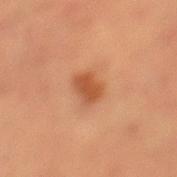<case>
<automated_metrics>
  <area_mm2_approx>6.0</area_mm2_approx>
  <eccentricity>0.7</eccentricity>
  <shape_asymmetry>0.2</shape_asymmetry>
  <cielab_L>47</cielab_L>
  <cielab_a>24</cielab_a>
  <cielab_b>35</cielab_b>
  <vs_skin_darker_L>10.0</vs_skin_darker_L>
  <vs_skin_contrast_norm>7.5</vs_skin_contrast_norm>
  <color_variation_0_10>2.0</color_variation_0_10>
</automated_metrics>
<image>
  <source>total-body photography crop</source>
  <field_of_view_mm>15</field_of_view_mm>
</image>
<lighting>cross-polarized</lighting>
<lesion_size>
  <long_diameter_mm_approx>3.0</long_diameter_mm_approx>
</lesion_size>
<site>left lower leg</site>
<patient>
  <sex>female</sex>
  <age_approx>60</age_approx>
</patient>
</case>